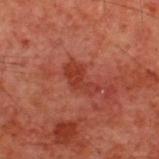Captured during whole-body skin photography for melanoma surveillance; the lesion was not biopsied. The lesion-visualizer software estimated a lesion area of about 6.5 mm², a shape eccentricity near 0.85, and a shape-asymmetry score of about 0.4 (0 = symmetric). It also reported an average lesion color of about L≈30 a*≈26 b*≈26 (CIELAB), a lesion–skin lightness drop of about 6, and a normalized border contrast of about 6.5. It also reported border irregularity of about 5 on a 0–10 scale, a within-lesion color-variation index near 1.5/10, and radial color variation of about 0.5. The analysis additionally found a classifier nevus-likeness of about 0/100 and lesion-presence confidence of about 100/100. A region of skin cropped from a whole-body photographic capture, roughly 15 mm wide. The lesion is located on the back. A male subject, in their 60s.